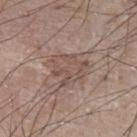Q: Is there a histopathology result?
A: no biopsy performed (imaged during a skin exam)
Q: Who is the patient?
A: male, in their mid- to late 70s
Q: What kind of image is this?
A: 15 mm crop, total-body photography
Q: What is the anatomic site?
A: the front of the torso